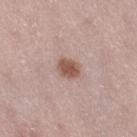Assessment: No biopsy was performed on this lesion — it was imaged during a full skin examination and was not determined to be concerning. Clinical summary: A roughly 15 mm field-of-view crop from a total-body skin photograph. Imaged with white-light lighting. An algorithmic analysis of the crop reported a mean CIELAB color near L≈54 a*≈20 b*≈26 and roughly 13 lightness units darker than nearby skin. The software also gave border irregularity of about 1 on a 0–10 scale, a color-variation rating of about 2/10, and a peripheral color-asymmetry measure near 0.5. A female patient, aged 38–42. Longest diameter approximately 3 mm. Located on the right thigh.